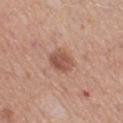The lesion was photographed on a routine skin check and not biopsied; there is no pathology result.
A female patient aged approximately 65.
On the left lower leg.
This image is a 15 mm lesion crop taken from a total-body photograph.